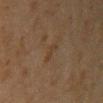Part of a total-body skin-imaging series; this lesion was reviewed on a skin check and was not flagged for biopsy.